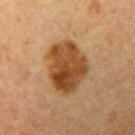This lesion was catalogued during total-body skin photography and was not selected for biopsy. From the left upper arm. The patient is a female in their mid- to late 50s. A lesion tile, about 15 mm wide, cut from a 3D total-body photograph.